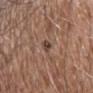<record>
  <biopsy_status>not biopsied; imaged during a skin examination</biopsy_status>
  <site>chest</site>
  <patient>
    <sex>male</sex>
    <age_approx>55</age_approx>
  </patient>
  <image>
    <source>total-body photography crop</source>
    <field_of_view_mm>15</field_of_view_mm>
  </image>
  <lesion_size>
    <long_diameter_mm_approx>2.5</long_diameter_mm_approx>
  </lesion_size>
</record>